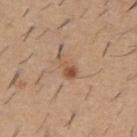Impression:
This lesion was catalogued during total-body skin photography and was not selected for biopsy.
Background:
Located on the back. The subject is a male in their 60s. The tile uses white-light illumination. A lesion tile, about 15 mm wide, cut from a 3D total-body photograph. Automated tile analysis of the lesion measured a lesion color around L≈53 a*≈20 b*≈32 in CIELAB and about 10 CIELAB-L* units darker than the surrounding skin. And it measured a border-irregularity rating of about 6/10, a within-lesion color-variation index near 2.5/10, and radial color variation of about 0.5. The analysis additionally found a nevus-likeness score of about 60/100 and lesion-presence confidence of about 100/100.